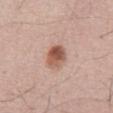biopsy_status: not biopsied; imaged during a skin examination
patient:
  sex: male
  age_approx: 60
image:
  source: total-body photography crop
  field_of_view_mm: 15
lighting: white-light
site: abdomen